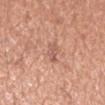Impression:
Part of a total-body skin-imaging series; this lesion was reviewed on a skin check and was not flagged for biopsy.
Clinical summary:
A close-up tile cropped from a whole-body skin photograph, about 15 mm across. The subject is a male aged approximately 55. The lesion is on the right forearm.